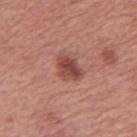Q: Was a biopsy performed?
A: total-body-photography surveillance lesion; no biopsy
Q: Lesion size?
A: ~3.5 mm (longest diameter)
Q: Patient demographics?
A: male, aged around 65
Q: How was this image acquired?
A: 15 mm crop, total-body photography
Q: What is the anatomic site?
A: the mid back
Q: Illumination type?
A: white-light illumination